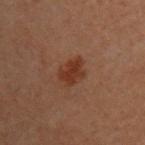No biopsy was performed on this lesion — it was imaged during a full skin examination and was not determined to be concerning. Automated image analysis of the tile measured a footprint of about 6 mm² and an outline eccentricity of about 0.7 (0 = round, 1 = elongated). It also reported a mean CIELAB color near L≈27 a*≈19 b*≈25 and about 7 CIELAB-L* units darker than the surrounding skin. The software also gave internal color variation of about 2 on a 0–10 scale and radial color variation of about 0.5. From the back. A lesion tile, about 15 mm wide, cut from a 3D total-body photograph. About 3.5 mm across. Imaged with cross-polarized lighting. A female patient, approximately 30 years of age.